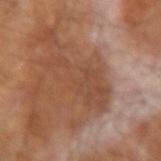The lesion was tiled from a total-body skin photograph and was not biopsied.
A 15 mm crop from a total-body photograph taken for skin-cancer surveillance.
A male patient roughly 65 years of age.
Imaged with cross-polarized lighting.
Approximately 7.5 mm at its widest.
Automated image analysis of the tile measured a footprint of about 19 mm², an outline eccentricity of about 0.9 (0 = round, 1 = elongated), and two-axis asymmetry of about 0.35.
From the right forearm.